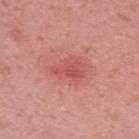  biopsy_status: not biopsied; imaged during a skin examination
  image:
    source: total-body photography crop
    field_of_view_mm: 15
  lesion_size:
    long_diameter_mm_approx: 4.0
  patient:
    sex: female
    age_approx: 40
  site: upper back
  automated_metrics:
    vs_skin_contrast_norm: 5.5
    border_irregularity_0_10: 5.0
    color_variation_0_10: 2.5
    peripheral_color_asymmetry: 1.0
  lighting: white-light